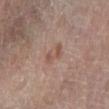Imaged during a routine full-body skin examination; the lesion was not biopsied and no histopathology is available.
The subject is a female approximately 75 years of age.
The lesion is on the right lower leg.
A close-up tile cropped from a whole-body skin photograph, about 15 mm across.